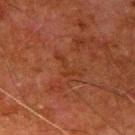Clinical impression: The lesion was tiled from a total-body skin photograph and was not biopsied. Clinical summary: A close-up tile cropped from a whole-body skin photograph, about 15 mm across. Imaged with cross-polarized lighting. A male patient, aged approximately 80. The lesion's longest dimension is about 4 mm. On the right upper arm.